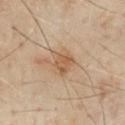Assessment:
Imaged during a routine full-body skin examination; the lesion was not biopsied and no histopathology is available.